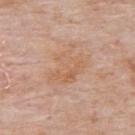The lesion was tiled from a total-body skin photograph and was not biopsied. The lesion-visualizer software estimated a nevus-likeness score of about 0/100 and lesion-presence confidence of about 100/100. The tile uses white-light illumination. Located on the upper back. A male patient, in their mid-70s. About 5.5 mm across. Cropped from a whole-body photographic skin survey; the tile spans about 15 mm.